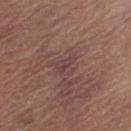follow-up: no biopsy performed (imaged during a skin exam) | lighting: white-light | anatomic site: the left thigh | imaging modality: ~15 mm tile from a whole-body skin photo | diameter: ~2.5 mm (longest diameter) | patient: female, aged 68–72.